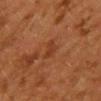notes = catalogued during a skin exam; not biopsied
lesion size = about 2.5 mm
site = the mid back
automated lesion analysis = a mean CIELAB color near L≈31 a*≈22 b*≈29 and a normalized border contrast of about 5.5
acquisition = ~15 mm crop, total-body skin-cancer survey
patient = female, aged 48 to 52
lighting = cross-polarized illumination